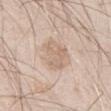{
  "lesion_size": {
    "long_diameter_mm_approx": 4.5
  },
  "site": "right thigh",
  "automated_metrics": {
    "vs_skin_darker_L": 8.0
  },
  "image": {
    "source": "total-body photography crop",
    "field_of_view_mm": 15
  },
  "patient": {
    "sex": "male",
    "age_approx": 65
  },
  "lighting": "white-light"
}